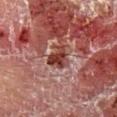This lesion was catalogued during total-body skin photography and was not selected for biopsy. Longest diameter approximately 3 mm. Automated image analysis of the tile measured a shape eccentricity near 0.6 and a shape-asymmetry score of about 0.45 (0 = symmetric). The analysis additionally found a mean CIELAB color near L≈34 a*≈25 b*≈23, a lesion–skin lightness drop of about 12, and a normalized border contrast of about 10.5. And it measured internal color variation of about 3.5 on a 0–10 scale and peripheral color asymmetry of about 1. A 15 mm close-up tile from a total-body photography series done for melanoma screening. The lesion is on the right lower leg. This is a cross-polarized tile. A male subject aged around 55.